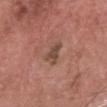This lesion was catalogued during total-body skin photography and was not selected for biopsy. A lesion tile, about 15 mm wide, cut from a 3D total-body photograph. The lesion's longest dimension is about 3 mm. The subject is a male aged approximately 65. The total-body-photography lesion software estimated a mean CIELAB color near L≈46 a*≈19 b*≈26. It also reported a classifier nevus-likeness of about 0/100 and a detector confidence of about 100 out of 100 that the crop contains a lesion. The lesion is on the head or neck. This is a white-light tile.